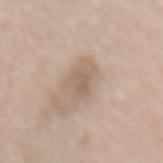image source=~15 mm crop, total-body skin-cancer survey
lesion size=about 4.5 mm
anatomic site=the lower back
tile lighting=white-light illumination
subject=female, aged 73–77